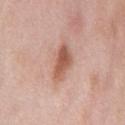{"biopsy_status": "not biopsied; imaged during a skin examination", "site": "chest", "lighting": "white-light", "lesion_size": {"long_diameter_mm_approx": 4.5}, "patient": {"sex": "male", "age_approx": 55}, "image": {"source": "total-body photography crop", "field_of_view_mm": 15}}